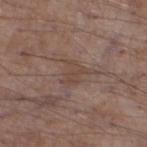Assessment: The lesion was tiled from a total-body skin photograph and was not biopsied. Background: From the left lower leg. The tile uses white-light illumination. Automated image analysis of the tile measured a footprint of about 5.5 mm², an outline eccentricity of about 0.6 (0 = round, 1 = elongated), and a symmetry-axis asymmetry near 0.4. The analysis additionally found a within-lesion color-variation index near 1.5/10 and a peripheral color-asymmetry measure near 0.5. It also reported a classifier nevus-likeness of about 0/100. A male patient, aged 53 to 57. A roughly 15 mm field-of-view crop from a total-body skin photograph. Measured at roughly 3.5 mm in maximum diameter.